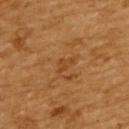notes: no biopsy performed (imaged during a skin exam)
TBP lesion metrics: an area of roughly 2.5 mm², an eccentricity of roughly 0.8, and a shape-asymmetry score of about 0.45 (0 = symmetric); internal color variation of about 0.5 on a 0–10 scale and radial color variation of about 0
illumination: cross-polarized
subject: female, roughly 55 years of age
imaging modality: total-body-photography crop, ~15 mm field of view
body site: the back